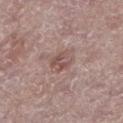Impression: Imaged during a routine full-body skin examination; the lesion was not biopsied and no histopathology is available. Background: The lesion is located on the left lower leg. A female patient in their mid- to late 60s. A 15 mm close-up tile from a total-body photography series done for melanoma screening. Measured at roughly 3 mm in maximum diameter.